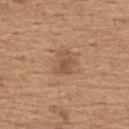Findings:
- biopsy status — no biopsy performed (imaged during a skin exam)
- anatomic site — the upper back
- patient — male, in their mid-60s
- acquisition — total-body-photography crop, ~15 mm field of view